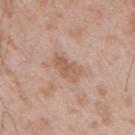Q: Is there a histopathology result?
A: total-body-photography surveillance lesion; no biopsy
Q: Patient demographics?
A: male, about 50 years old
Q: What is the imaging modality?
A: total-body-photography crop, ~15 mm field of view
Q: Lesion location?
A: the right upper arm